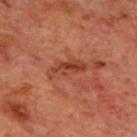Clinical impression:
This lesion was catalogued during total-body skin photography and was not selected for biopsy.
Acquisition and patient details:
A 15 mm close-up tile from a total-body photography series done for melanoma screening. The total-body-photography lesion software estimated a footprint of about 6.5 mm² and two-axis asymmetry of about 0.35. The analysis additionally found a mean CIELAB color near L≈41 a*≈29 b*≈33, a lesion–skin lightness drop of about 9, and a normalized border contrast of about 7. The software also gave an automated nevus-likeness rating near 0 out of 100 and a detector confidence of about 100 out of 100 that the crop contains a lesion. A male subject about 70 years old. Located on the upper back. The recorded lesion diameter is about 4.5 mm. Captured under cross-polarized illumination.